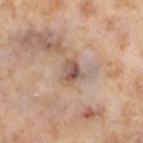Case summary:
– follow-up: total-body-photography surveillance lesion; no biopsy
– size: ≈2.5 mm
– image-analysis metrics: a footprint of about 5 mm² and a shape eccentricity near 0.5; an average lesion color of about L≈55 a*≈18 b*≈26 (CIELAB), roughly 11 lightness units darker than nearby skin, and a normalized border contrast of about 8
– subject: female, aged approximately 55
– image: total-body-photography crop, ~15 mm field of view
– lighting: cross-polarized
– site: the leg